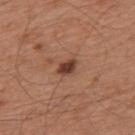No biopsy was performed on this lesion — it was imaged during a full skin examination and was not determined to be concerning. The tile uses white-light illumination. A roughly 15 mm field-of-view crop from a total-body skin photograph. A male subject, approximately 65 years of age. Located on the upper back. About 2.5 mm across.